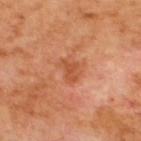Impression: No biopsy was performed on this lesion — it was imaged during a full skin examination and was not determined to be concerning. Image and clinical context: A male subject aged approximately 70. Approximately 2.5 mm at its widest. The tile uses cross-polarized illumination. A 15 mm close-up extracted from a 3D total-body photography capture. Located on the upper back.